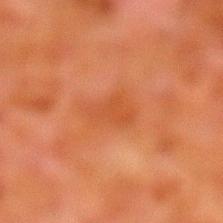Q: Was a biopsy performed?
A: total-body-photography surveillance lesion; no biopsy
Q: What lighting was used for the tile?
A: cross-polarized
Q: Patient demographics?
A: male, aged 78 to 82
Q: Lesion size?
A: ~4 mm (longest diameter)
Q: What is the anatomic site?
A: the leg
Q: What is the imaging modality?
A: ~15 mm crop, total-body skin-cancer survey